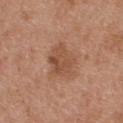Findings:
- notes — catalogued during a skin exam; not biopsied
- size — ≈4 mm
- subject — male, about 30 years old
- location — the upper back
- illumination — white-light illumination
- acquisition — ~15 mm tile from a whole-body skin photo